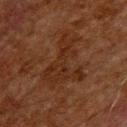{"biopsy_status": "not biopsied; imaged during a skin examination", "site": "upper back", "lighting": "cross-polarized", "image": {"source": "total-body photography crop", "field_of_view_mm": 15}, "automated_metrics": {"border_irregularity_0_10": 9.5, "nevus_likeness_0_100": 0}, "lesion_size": {"long_diameter_mm_approx": 7.0}, "patient": {"sex": "male", "age_approx": 60}}